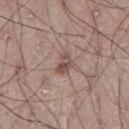follow-up: imaged on a skin check; not biopsied | site: the right thigh | imaging modality: 15 mm crop, total-body photography | lighting: white-light | subject: male, aged 18–22 | TBP lesion metrics: an area of roughly 4 mm², an outline eccentricity of about 0.7 (0 = round, 1 = elongated), and a symmetry-axis asymmetry near 0.3; a color-variation rating of about 5/10 and a peripheral color-asymmetry measure near 2.